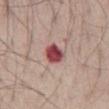Captured during whole-body skin photography for melanoma surveillance; the lesion was not biopsied. From the abdomen. The recorded lesion diameter is about 2.5 mm. A male patient, aged around 55. The tile uses white-light illumination. Automated image analysis of the tile measured an eccentricity of roughly 0.45 and a shape-asymmetry score of about 0.25 (0 = symmetric). And it measured a lesion color around L≈47 a*≈31 b*≈21 in CIELAB, roughly 18 lightness units darker than nearby skin, and a lesion-to-skin contrast of about 12.5 (normalized; higher = more distinct). It also reported radial color variation of about 1.5. The software also gave an automated nevus-likeness rating near 0 out of 100 and a lesion-detection confidence of about 100/100. A lesion tile, about 15 mm wide, cut from a 3D total-body photograph.